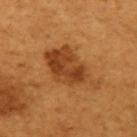Q: Was this lesion biopsied?
A: no biopsy performed (imaged during a skin exam)
Q: How large is the lesion?
A: ≈7 mm
Q: Lesion location?
A: the arm
Q: Automated lesion metrics?
A: a nevus-likeness score of about 60/100
Q: What is the imaging modality?
A: ~15 mm tile from a whole-body skin photo
Q: Patient demographics?
A: male, approximately 65 years of age
Q: Illumination type?
A: cross-polarized